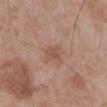Impression:
No biopsy was performed on this lesion — it was imaged during a full skin examination and was not determined to be concerning.
Image and clinical context:
The total-body-photography lesion software estimated a footprint of about 3.5 mm² and a shape-asymmetry score of about 0.3 (0 = symmetric). And it measured roughly 7 lightness units darker than nearby skin and a lesion-to-skin contrast of about 5 (normalized; higher = more distinct). The lesion is located on the front of the torso. Longest diameter approximately 2.5 mm. Captured under white-light illumination. A roughly 15 mm field-of-view crop from a total-body skin photograph. The subject is a male in their 70s.